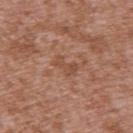<case>
<patient>
  <sex>male</sex>
  <age_approx>45</age_approx>
</patient>
<image>
  <source>total-body photography crop</source>
  <field_of_view_mm>15</field_of_view_mm>
</image>
<lighting>white-light</lighting>
<site>upper back</site>
</case>